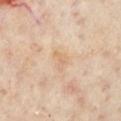{"lesion_size": {"long_diameter_mm_approx": 2.5}, "patient": {"sex": "female", "age_approx": 60}, "automated_metrics": {"cielab_L": 69, "cielab_a": 16, "cielab_b": 34, "vs_skin_darker_L": 6.0, "vs_skin_contrast_norm": 4.5}, "image": {"source": "total-body photography crop", "field_of_view_mm": 15}, "site": "front of the torso", "lighting": "cross-polarized"}